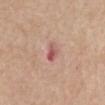Notes:
* biopsy status: imaged on a skin check; not biopsied
* imaging modality: ~15 mm tile from a whole-body skin photo
* subject: female, aged 63–67
* lesion size: ≈3 mm
* site: the abdomen